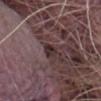This lesion was catalogued during total-body skin photography and was not selected for biopsy.
Measured at roughly 2.5 mm in maximum diameter.
Imaged with white-light lighting.
The subject is a male aged 63 to 67.
Automated image analysis of the tile measured an average lesion color of about L≈30 a*≈17 b*≈14 (CIELAB) and a normalized border contrast of about 9. It also reported a border-irregularity rating of about 2.5/10, a within-lesion color-variation index near 4.5/10, and radial color variation of about 2. The analysis additionally found a nevus-likeness score of about 5/100 and a detector confidence of about 75 out of 100 that the crop contains a lesion.
From the abdomen.
A roughly 15 mm field-of-view crop from a total-body skin photograph.